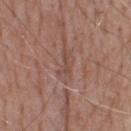This lesion was catalogued during total-body skin photography and was not selected for biopsy. The patient is a male aged 63 to 67. The lesion is located on the upper back. Cropped from a whole-body photographic skin survey; the tile spans about 15 mm.